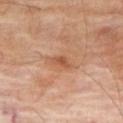Captured during whole-body skin photography for melanoma surveillance; the lesion was not biopsied.
Located on the right thigh.
A male patient about 70 years old.
A 15 mm crop from a total-body photograph taken for skin-cancer surveillance.